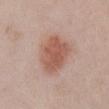Findings:
• location — the abdomen
• image-analysis metrics — a footprint of about 17 mm²; a lesion–skin lightness drop of about 11 and a normalized border contrast of about 7.5; a border-irregularity rating of about 2/10, a color-variation rating of about 3/10, and a peripheral color-asymmetry measure near 1
• image source — ~15 mm tile from a whole-body skin photo
• lesion size — ~5.5 mm (longest diameter)
• illumination — white-light
• subject — female, approximately 75 years of age